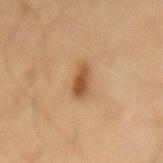The lesion was tiled from a total-body skin photograph and was not biopsied. The total-body-photography lesion software estimated lesion-presence confidence of about 100/100. Longest diameter approximately 3.5 mm. Imaged with cross-polarized lighting. The lesion is on the back. Cropped from a whole-body photographic skin survey; the tile spans about 15 mm. A male patient roughly 65 years of age.